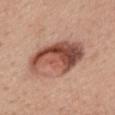Assessment: The lesion was photographed on a routine skin check and not biopsied; there is no pathology result. Clinical summary: Cropped from a whole-body photographic skin survey; the tile spans about 15 mm. Automated image analysis of the tile measured border irregularity of about 3 on a 0–10 scale and a color-variation rating of about 10/10. The software also gave a nevus-likeness score of about 95/100 and lesion-presence confidence of about 100/100. A female subject roughly 55 years of age. Located on the back. This is a white-light tile.